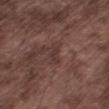Assessment: The lesion was photographed on a routine skin check and not biopsied; there is no pathology result. Context: About 2.5 mm across. Automated image analysis of the tile measured an area of roughly 3 mm², an outline eccentricity of about 0.9 (0 = round, 1 = elongated), and a symmetry-axis asymmetry near 0.4. The software also gave a border-irregularity index near 4/10, internal color variation of about 0 on a 0–10 scale, and radial color variation of about 0. Located on the leg. Cropped from a whole-body photographic skin survey; the tile spans about 15 mm. The subject is a male about 75 years old.